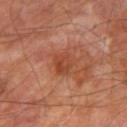Assessment: Part of a total-body skin-imaging series; this lesion was reviewed on a skin check and was not flagged for biopsy. Background: The total-body-photography lesion software estimated an area of roughly 5 mm², an eccentricity of roughly 0.5, and a symmetry-axis asymmetry near 0.2. The software also gave a border-irregularity rating of about 2/10. Located on the right thigh. Captured under cross-polarized illumination. A male subject, approximately 70 years of age. Cropped from a total-body skin-imaging series; the visible field is about 15 mm.